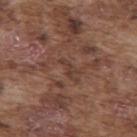Impression: Captured during whole-body skin photography for melanoma surveillance; the lesion was not biopsied.